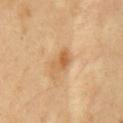Findings:
- notes — imaged on a skin check; not biopsied
- image source — ~15 mm tile from a whole-body skin photo
- illumination — cross-polarized illumination
- body site — the chest
- subject — male, aged around 50